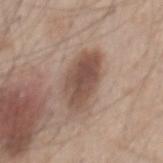biopsy status: catalogued during a skin exam; not biopsied | subject: male, roughly 45 years of age | location: the mid back | illumination: white-light | imaging modality: total-body-photography crop, ~15 mm field of view.